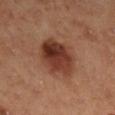{
  "biopsy_status": "not biopsied; imaged during a skin examination",
  "lighting": "cross-polarized",
  "lesion_size": {
    "long_diameter_mm_approx": 5.0
  },
  "automated_metrics": {
    "area_mm2_approx": 17.0,
    "eccentricity": 0.45,
    "color_variation_0_10": 8.0
  },
  "image": {
    "source": "total-body photography crop",
    "field_of_view_mm": 15
  },
  "site": "leg",
  "patient": {
    "sex": "female",
    "age_approx": 50
  }
}